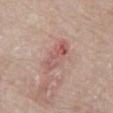Impression:
Captured during whole-body skin photography for melanoma surveillance; the lesion was not biopsied.
Background:
The lesion is on the abdomen. The tile uses white-light illumination. A male subject aged approximately 80. Cropped from a total-body skin-imaging series; the visible field is about 15 mm. The lesion-visualizer software estimated a footprint of about 9 mm², an eccentricity of roughly 0.85, and a symmetry-axis asymmetry near 0.25. The software also gave an automated nevus-likeness rating near 5 out of 100 and a detector confidence of about 100 out of 100 that the crop contains a lesion.